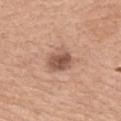Captured during whole-body skin photography for melanoma surveillance; the lesion was not biopsied.
The recorded lesion diameter is about 3.5 mm.
On the upper back.
A female subject about 60 years old.
This is a white-light tile.
A 15 mm crop from a total-body photograph taken for skin-cancer surveillance.